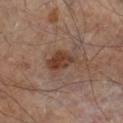<record>
  <biopsy_status>not biopsied; imaged during a skin examination</biopsy_status>
  <automated_metrics>
    <area_mm2_approx>7.5</area_mm2_approx>
    <eccentricity>0.75</eccentricity>
    <vs_skin_darker_L>9.0</vs_skin_darker_L>
    <border_irregularity_0_10>2.5</border_irregularity_0_10>
    <peripheral_color_asymmetry>1.5</peripheral_color_asymmetry>
    <lesion_detection_confidence_0_100>100</lesion_detection_confidence_0_100>
  </automated_metrics>
  <lighting>cross-polarized</lighting>
  <lesion_size>
    <long_diameter_mm_approx>3.5</long_diameter_mm_approx>
  </lesion_size>
  <site>right lower leg</site>
  <image>
    <source>total-body photography crop</source>
    <field_of_view_mm>15</field_of_view_mm>
  </image>
  <patient>
    <sex>male</sex>
    <age_approx>65</age_approx>
  </patient>
</record>